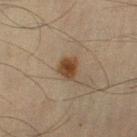Assessment: Imaged during a routine full-body skin examination; the lesion was not biopsied and no histopathology is available. Clinical summary: A region of skin cropped from a whole-body photographic capture, roughly 15 mm wide. From the right upper arm. The total-body-photography lesion software estimated an average lesion color of about L≈34 a*≈15 b*≈27 (CIELAB). And it measured an automated nevus-likeness rating near 100 out of 100. Longest diameter approximately 2.5 mm. A male subject, roughly 70 years of age. Captured under cross-polarized illumination.